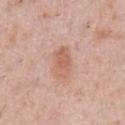* lesion size — ~3.5 mm (longest diameter)
* subject — male, aged approximately 40
* acquisition — ~15 mm tile from a whole-body skin photo
* location — the abdomen